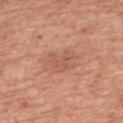Case summary:
• follow-up: total-body-photography surveillance lesion; no biopsy
• subject: male, in their mid-60s
• lesion size: about 5 mm
• lighting: white-light illumination
• automated metrics: an average lesion color of about L≈57 a*≈24 b*≈32 (CIELAB) and a lesion-to-skin contrast of about 4.5 (normalized; higher = more distinct); a border-irregularity rating of about 2.5/10, a color-variation rating of about 2.5/10, and radial color variation of about 1
• image source: total-body-photography crop, ~15 mm field of view
• site: the arm